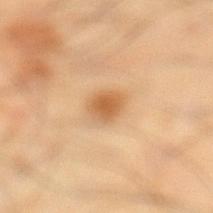<lesion>
  <biopsy_status>not biopsied; imaged during a skin examination</biopsy_status>
  <patient>
    <sex>male</sex>
    <age_approx>40</age_approx>
  </patient>
  <lighting>cross-polarized</lighting>
  <image>
    <source>total-body photography crop</source>
    <field_of_view_mm>15</field_of_view_mm>
  </image>
  <lesion_size>
    <long_diameter_mm_approx>2.5</long_diameter_mm_approx>
  </lesion_size>
  <site>left lower leg</site>
</lesion>